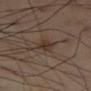{"biopsy_status": "not biopsied; imaged during a skin examination", "patient": {"sex": "male", "age_approx": 55}, "site": "left thigh", "lesion_size": {"long_diameter_mm_approx": 3.0}, "image": {"source": "total-body photography crop", "field_of_view_mm": 15}, "lighting": "cross-polarized"}